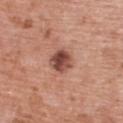The lesion was tiled from a total-body skin photograph and was not biopsied. From the upper back. The tile uses white-light illumination. The total-body-photography lesion software estimated border irregularity of about 2 on a 0–10 scale, a color-variation rating of about 6.5/10, and radial color variation of about 2.5. A 15 mm crop from a total-body photograph taken for skin-cancer surveillance. Longest diameter approximately 3 mm. A female subject about 60 years old.